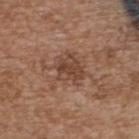About 3.5 mm across. The lesion is on the upper back. The subject is a female aged 63–67. Cropped from a total-body skin-imaging series; the visible field is about 15 mm. The tile uses white-light illumination. The total-body-photography lesion software estimated a border-irregularity index near 3.5/10 and internal color variation of about 3.5 on a 0–10 scale. The analysis additionally found a classifier nevus-likeness of about 0/100 and a detector confidence of about 100 out of 100 that the crop contains a lesion.